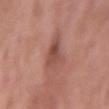image: ~15 mm tile from a whole-body skin photo
automated metrics: a lesion area of about 6.5 mm², a shape eccentricity near 0.55, and a symmetry-axis asymmetry near 0.35; a classifier nevus-likeness of about 0/100
patient: female, aged 68 to 72
lesion size: ~3.5 mm (longest diameter)
lighting: white-light
site: the left upper arm